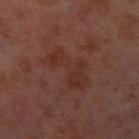* site — the left upper arm
* patient — male, aged around 30
* image — total-body-photography crop, ~15 mm field of view
* size — about 6.5 mm
* automated lesion analysis — roughly 4 lightness units darker than nearby skin and a normalized border contrast of about 5; border irregularity of about 7 on a 0–10 scale, a within-lesion color-variation index near 3/10, and a peripheral color-asymmetry measure near 1
* lighting — cross-polarized illumination